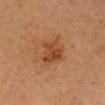Q: Was this lesion biopsied?
A: catalogued during a skin exam; not biopsied
Q: Lesion size?
A: ~3.5 mm (longest diameter)
Q: What are the patient's age and sex?
A: male, roughly 65 years of age
Q: How was this image acquired?
A: ~15 mm crop, total-body skin-cancer survey
Q: What is the anatomic site?
A: the head or neck
Q: What lighting was used for the tile?
A: cross-polarized illumination